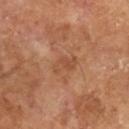follow-up=imaged on a skin check; not biopsied | diameter=about 3.5 mm | tile lighting=cross-polarized | patient=male, roughly 65 years of age | automated lesion analysis=a footprint of about 4.5 mm² and an eccentricity of roughly 0.85; a lesion–skin lightness drop of about 7 and a normalized border contrast of about 5; a border-irregularity rating of about 3.5/10, internal color variation of about 2.5 on a 0–10 scale, and peripheral color asymmetry of about 1 | acquisition=~15 mm tile from a whole-body skin photo.